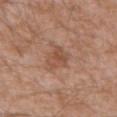follow-up: imaged on a skin check; not biopsied
patient: male, in their 60s
lighting: white-light
imaging modality: total-body-photography crop, ~15 mm field of view
location: the mid back
lesion size: about 3 mm
image-analysis metrics: a lesion area of about 5 mm², an outline eccentricity of about 0.6 (0 = round, 1 = elongated), and a shape-asymmetry score of about 0.4 (0 = symmetric); about 8 CIELAB-L* units darker than the surrounding skin and a normalized border contrast of about 6; a classifier nevus-likeness of about 0/100 and a lesion-detection confidence of about 100/100